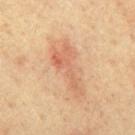The lesion was photographed on a routine skin check and not biopsied; there is no pathology result. A male patient, in their mid- to late 60s. The lesion is on the mid back. A roughly 15 mm field-of-view crop from a total-body skin photograph.